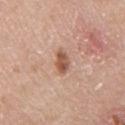biopsy_status: not biopsied; imaged during a skin examination
site: front of the torso
patient:
  sex: male
  age_approx: 60
automated_metrics:
  eccentricity: 0.8
  shape_asymmetry: 0.2
  lesion_detection_confidence_0_100: 100
image:
  source: total-body photography crop
  field_of_view_mm: 15
lesion_size:
  long_diameter_mm_approx: 3.0
lighting: white-light